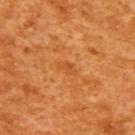The lesion was tiled from a total-body skin photograph and was not biopsied.
The recorded lesion diameter is about 2.5 mm.
A region of skin cropped from a whole-body photographic capture, roughly 15 mm wide.
The total-body-photography lesion software estimated a lesion area of about 2.5 mm² and a shape-asymmetry score of about 0.3 (0 = symmetric). The software also gave a normalized border contrast of about 4.5. The software also gave border irregularity of about 3 on a 0–10 scale and radial color variation of about 0. The software also gave a nevus-likeness score of about 0/100 and a detector confidence of about 100 out of 100 that the crop contains a lesion.
A female subject, aged around 55.
Located on the arm.
Imaged with cross-polarized lighting.